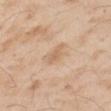Part of a total-body skin-imaging series; this lesion was reviewed on a skin check and was not flagged for biopsy. A roughly 15 mm field-of-view crop from a total-body skin photograph. The lesion is on the left upper arm. Longest diameter approximately 2.5 mm. Automated image analysis of the tile measured a lesion area of about 3 mm², a shape eccentricity near 0.85, and a shape-asymmetry score of about 0.25 (0 = symmetric). The analysis additionally found a lesion color around L≈63 a*≈18 b*≈33 in CIELAB and about 8 CIELAB-L* units darker than the surrounding skin. The analysis additionally found a classifier nevus-likeness of about 0/100. A male subject, in their mid- to late 50s. This is a cross-polarized tile.